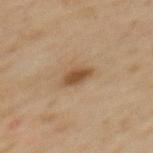Findings:
* workup: catalogued during a skin exam; not biopsied
* subject: female, roughly 60 years of age
* image source: ~15 mm tile from a whole-body skin photo
* lesion size: ~3 mm (longest diameter)
* location: the mid back
* tile lighting: cross-polarized